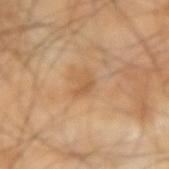Q: Was a biopsy performed?
A: imaged on a skin check; not biopsied